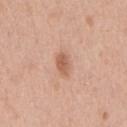Clinical impression:
Part of a total-body skin-imaging series; this lesion was reviewed on a skin check and was not flagged for biopsy.
Image and clinical context:
On the chest. A lesion tile, about 15 mm wide, cut from a 3D total-body photograph. A male patient, in their mid-50s.